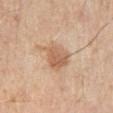Notes:
- follow-up · total-body-photography surveillance lesion; no biopsy
- acquisition · 15 mm crop, total-body photography
- anatomic site · the leg
- patient · male, approximately 60 years of age
- tile lighting · cross-polarized
- diameter · about 3.5 mm